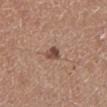| feature | finding |
|---|---|
| workup | catalogued during a skin exam; not biopsied |
| anatomic site | the left lower leg |
| image | 15 mm crop, total-body photography |
| patient | male, roughly 50 years of age |